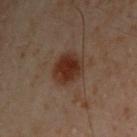| key | value |
|---|---|
| biopsy status | imaged on a skin check; not biopsied |
| lesion size | ≈3.5 mm |
| subject | male, roughly 50 years of age |
| location | the arm |
| image | 15 mm crop, total-body photography |
| image-analysis metrics | a footprint of about 11 mm², an eccentricity of roughly 0.45, and two-axis asymmetry of about 0.15 |
| lighting | cross-polarized |